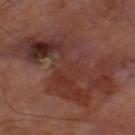follow-up: catalogued during a skin exam; not biopsied
image: total-body-photography crop, ~15 mm field of view
site: the left thigh
patient: male, in their 70s